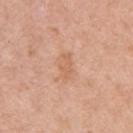| key | value |
|---|---|
| subject | female, roughly 40 years of age |
| tile lighting | white-light |
| anatomic site | the left upper arm |
| image source | 15 mm crop, total-body photography |